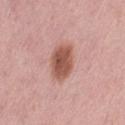Part of a total-body skin-imaging series; this lesion was reviewed on a skin check and was not flagged for biopsy. Longest diameter approximately 4.5 mm. The patient is a female about 50 years old. Automated tile analysis of the lesion measured a footprint of about 11 mm², an eccentricity of roughly 0.8, and a symmetry-axis asymmetry near 0.2. And it measured a border-irregularity index near 2/10, a color-variation rating of about 3/10, and peripheral color asymmetry of about 1. Located on the left thigh. The tile uses white-light illumination. A 15 mm crop from a total-body photograph taken for skin-cancer surveillance.